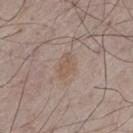biopsy status = catalogued during a skin exam; not biopsied | imaging modality = ~15 mm tile from a whole-body skin photo | patient = male, aged around 75 | body site = the left thigh | tile lighting = white-light illumination.